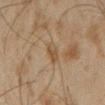Captured during whole-body skin photography for melanoma surveillance; the lesion was not biopsied.
A male subject approximately 45 years of age.
Located on the left forearm.
This image is a 15 mm lesion crop taken from a total-body photograph.
The lesion's longest dimension is about 2.5 mm.
The total-body-photography lesion software estimated a lesion area of about 4 mm², an eccentricity of roughly 0.7, and two-axis asymmetry of about 0.2.